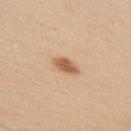A 15 mm close-up tile from a total-body photography series done for melanoma screening. The lesion is located on the upper back. A male patient, in their 30s.